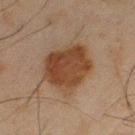follow-up: catalogued during a skin exam; not biopsied | location: the left thigh | subject: male, approximately 45 years of age | image-analysis metrics: a footprint of about 23 mm²; an automated nevus-likeness rating near 85 out of 100 | illumination: cross-polarized illumination | acquisition: total-body-photography crop, ~15 mm field of view.